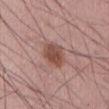<tbp_lesion>
<biopsy_status>not biopsied; imaged during a skin examination</biopsy_status>
<lesion_size>
  <long_diameter_mm_approx>3.0</long_diameter_mm_approx>
</lesion_size>
<patient>
  <sex>male</sex>
  <age_approx>55</age_approx>
</patient>
<lighting>white-light</lighting>
<site>abdomen</site>
<image>
  <source>total-body photography crop</source>
  <field_of_view_mm>15</field_of_view_mm>
</image>
</tbp_lesion>